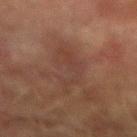No biopsy was performed on this lesion — it was imaged during a full skin examination and was not determined to be concerning. Located on the left forearm. A male subject, approximately 75 years of age. A 15 mm close-up tile from a total-body photography series done for melanoma screening.